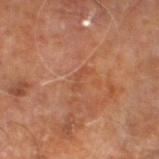Impression: The lesion was tiled from a total-body skin photograph and was not biopsied. Clinical summary: A lesion tile, about 15 mm wide, cut from a 3D total-body photograph. From the right leg. A male subject in their 60s.